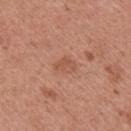Notes:
- notes · catalogued during a skin exam; not biopsied
- patient · male, aged 43–47
- anatomic site · the left upper arm
- lesion size · ~2.5 mm (longest diameter)
- tile lighting · white-light
- acquisition · total-body-photography crop, ~15 mm field of view